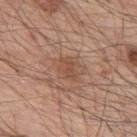{
  "biopsy_status": "not biopsied; imaged during a skin examination",
  "lighting": "white-light",
  "automated_metrics": {
    "cielab_L": 50,
    "cielab_a": 21,
    "cielab_b": 29,
    "vs_skin_contrast_norm": 6.0,
    "nevus_likeness_0_100": 10,
    "lesion_detection_confidence_0_100": 100
  },
  "patient": {
    "sex": "male",
    "age_approx": 55
  },
  "image": {
    "source": "total-body photography crop",
    "field_of_view_mm": 15
  },
  "site": "upper back"
}